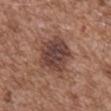Clinical impression: Recorded during total-body skin imaging; not selected for excision or biopsy. Background: This image is a 15 mm lesion crop taken from a total-body photograph. A male patient, about 75 years old. The lesion is located on the front of the torso.